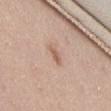Notes:
– follow-up: imaged on a skin check; not biopsied
– tile lighting: white-light
– size: ≈2.5 mm
– subject: female, in their mid-40s
– automated lesion analysis: an eccentricity of roughly 0.9 and a symmetry-axis asymmetry near 0.25; a lesion color around L≈60 a*≈19 b*≈28 in CIELAB and roughly 9 lightness units darker than nearby skin; a within-lesion color-variation index near 1/10 and peripheral color asymmetry of about 0
– acquisition: total-body-photography crop, ~15 mm field of view
– anatomic site: the lower back